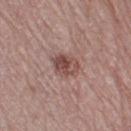The patient is a female about 65 years old. An algorithmic analysis of the crop reported a lesion color around L≈47 a*≈21 b*≈22 in CIELAB and a lesion-to-skin contrast of about 8 (normalized; higher = more distinct). The analysis additionally found border irregularity of about 1.5 on a 0–10 scale and internal color variation of about 6.5 on a 0–10 scale. Longest diameter approximately 3.5 mm. On the right thigh. Cropped from a whole-body photographic skin survey; the tile spans about 15 mm.